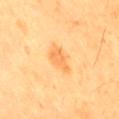Recorded during total-body skin imaging; not selected for excision or biopsy. On the leg. A 15 mm close-up extracted from a 3D total-body photography capture. Captured under cross-polarized illumination. A male subject, approximately 60 years of age. The total-body-photography lesion software estimated an outline eccentricity of about 0.8 (0 = round, 1 = elongated) and two-axis asymmetry of about 0.35. The analysis additionally found an average lesion color of about L≈70 a*≈26 b*≈49 (CIELAB) and a lesion–skin lightness drop of about 9. The analysis additionally found an automated nevus-likeness rating near 30 out of 100 and a detector confidence of about 100 out of 100 that the crop contains a lesion.